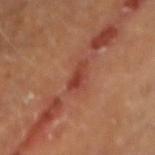workup=no biopsy performed (imaged during a skin exam) | patient=male, aged approximately 60 | imaging modality=15 mm crop, total-body photography | tile lighting=cross-polarized | diameter=about 3 mm | automated metrics=a footprint of about 3.5 mm², a shape eccentricity near 0.9, and a symmetry-axis asymmetry near 0.3; an average lesion color of about L≈39 a*≈28 b*≈30 (CIELAB) and a normalized lesion–skin contrast near 7; border irregularity of about 3.5 on a 0–10 scale, a within-lesion color-variation index near 0.5/10, and radial color variation of about 0; a classifier nevus-likeness of about 0/100 and lesion-presence confidence of about 100/100 | site=the right forearm.